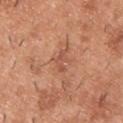Recorded during total-body skin imaging; not selected for excision or biopsy. A male patient aged approximately 40. About 2.5 mm across. Imaged with white-light lighting. The lesion is on the upper back. A 15 mm crop from a total-body photograph taken for skin-cancer surveillance.